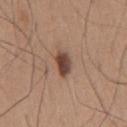workup — imaged on a skin check; not biopsied
site — the chest
patient — male, aged 63–67
acquisition — ~15 mm crop, total-body skin-cancer survey
lesion size — ~3.5 mm (longest diameter)
automated lesion analysis — roughly 15 lightness units darker than nearby skin and a lesion-to-skin contrast of about 11 (normalized; higher = more distinct); a nevus-likeness score of about 100/100 and a lesion-detection confidence of about 100/100